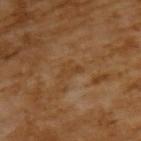Captured under cross-polarized illumination. A male subject about 65 years old. A lesion tile, about 15 mm wide, cut from a 3D total-body photograph.